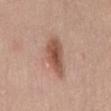Notes:
- follow-up: catalogued during a skin exam; not biopsied
- acquisition: ~15 mm crop, total-body skin-cancer survey
- site: the abdomen
- subject: male, aged approximately 55